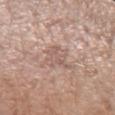  biopsy_status: not biopsied; imaged during a skin examination